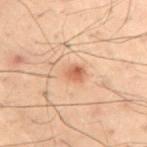Captured during whole-body skin photography for melanoma surveillance; the lesion was not biopsied.
The subject is a male in their 30s.
The tile uses cross-polarized illumination.
The lesion is located on the lower back.
Cropped from a total-body skin-imaging series; the visible field is about 15 mm.
The lesion's longest dimension is about 3 mm.
Automated tile analysis of the lesion measured a shape eccentricity near 0.7. It also reported a lesion color around L≈53 a*≈19 b*≈29 in CIELAB, roughly 7 lightness units darker than nearby skin, and a normalized border contrast of about 5.5. The software also gave an automated nevus-likeness rating near 85 out of 100 and a detector confidence of about 100 out of 100 that the crop contains a lesion.